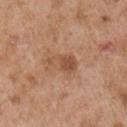lighting = white-light
lesion size = ≈4 mm
image-analysis metrics = an area of roughly 7 mm², an eccentricity of roughly 0.85, and two-axis asymmetry of about 0.3; an average lesion color of about L≈52 a*≈22 b*≈32 (CIELAB), about 9 CIELAB-L* units darker than the surrounding skin, and a normalized border contrast of about 6.5; an automated nevus-likeness rating near 10 out of 100 and lesion-presence confidence of about 100/100
anatomic site = the right upper arm
image source = ~15 mm tile from a whole-body skin photo
patient = male, aged 53 to 57